Captured under white-light illumination · the lesion is on the head or neck · about 2 mm across · Automated image analysis of the tile measured a lesion area of about 2 mm² and two-axis asymmetry of about 0.4. The analysis additionally found a mean CIELAB color near L≈44 a*≈22 b*≈24, about 10 CIELAB-L* units darker than the surrounding skin, and a normalized lesion–skin contrast near 7.5. It also reported a nevus-likeness score of about 40/100 and lesion-presence confidence of about 100/100 · a 15 mm close-up extracted from a 3D total-body photography capture · a male patient in their 30s.
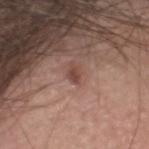{
  "diagnosis": {
    "histopathology": "dysplastic (Clark) nevus",
    "malignancy": "benign",
    "taxonomic_path": [
      "Benign",
      "Benign melanocytic proliferations",
      "Nevus",
      "Nevus, Atypical, Dysplastic, or Clark"
    ]
  }
}